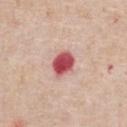Captured during whole-body skin photography for melanoma surveillance; the lesion was not biopsied. About 3.5 mm across. This image is a 15 mm lesion crop taken from a total-body photograph. The patient is a male aged 58 to 62. The lesion is on the chest. Automated tile analysis of the lesion measured a footprint of about 7 mm² and an eccentricity of roughly 0.65. It also reported an average lesion color of about L≈54 a*≈35 b*≈24 (CIELAB), roughly 19 lightness units darker than nearby skin, and a normalized lesion–skin contrast near 12. Captured under white-light illumination.